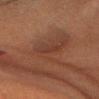Clinical impression:
Imaged during a routine full-body skin examination; the lesion was not biopsied and no histopathology is available.
Context:
The total-body-photography lesion software estimated an average lesion color of about L≈29 a*≈18 b*≈23 (CIELAB) and a lesion–skin lightness drop of about 5. It also reported a border-irregularity rating of about 4/10, a within-lesion color-variation index near 1/10, and radial color variation of about 0.5. The software also gave an automated nevus-likeness rating near 0 out of 100. From the head or neck. A 15 mm crop from a total-body photograph taken for skin-cancer surveillance. This is a cross-polarized tile. A female patient aged 48–52.